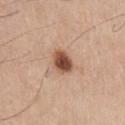<lesion>
  <biopsy_status>not biopsied; imaged during a skin examination</biopsy_status>
  <patient>
    <sex>male</sex>
    <age_approx>65</age_approx>
  </patient>
  <image>
    <source>total-body photography crop</source>
    <field_of_view_mm>15</field_of_view_mm>
  </image>
  <site>chest</site>
  <lighting>white-light</lighting>
  <lesion_size>
    <long_diameter_mm_approx>3.5</long_diameter_mm_approx>
  </lesion_size>
</lesion>